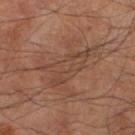<lesion>
  <biopsy_status>not biopsied; imaged during a skin examination</biopsy_status>
  <patient>
    <sex>male</sex>
    <age_approx>65</age_approx>
  </patient>
  <site>left lower leg</site>
  <image>
    <source>total-body photography crop</source>
    <field_of_view_mm>15</field_of_view_mm>
  </image>
</lesion>